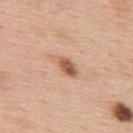Q: Was this lesion biopsied?
A: catalogued during a skin exam; not biopsied
Q: What is the imaging modality?
A: ~15 mm tile from a whole-body skin photo
Q: Patient demographics?
A: male, aged 58 to 62
Q: Lesion location?
A: the upper back
Q: Automated lesion metrics?
A: an area of roughly 5.5 mm² and an outline eccentricity of about 0.85 (0 = round, 1 = elongated); roughly 12 lightness units darker than nearby skin and a normalized border contrast of about 8; a classifier nevus-likeness of about 80/100